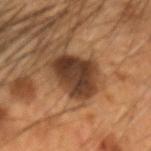<record>
  <biopsy_status>not biopsied; imaged during a skin examination</biopsy_status>
  <lighting>cross-polarized</lighting>
  <image>
    <source>total-body photography crop</source>
    <field_of_view_mm>15</field_of_view_mm>
  </image>
  <lesion_size>
    <long_diameter_mm_approx>5.0</long_diameter_mm_approx>
  </lesion_size>
  <automated_metrics>
    <eccentricity>0.6</eccentricity>
    <shape_asymmetry>0.25</shape_asymmetry>
  </automated_metrics>
  <patient>
    <sex>male</sex>
    <age_approx>55</age_approx>
  </patient>
  <site>head or neck</site>
</record>